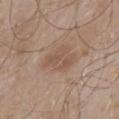<tbp_lesion>
  <biopsy_status>not biopsied; imaged during a skin examination</biopsy_status>
  <automated_metrics>
    <eccentricity>0.75</eccentricity>
    <shape_asymmetry>0.3</shape_asymmetry>
    <border_irregularity_0_10>3.5</border_irregularity_0_10>
    <color_variation_0_10>2.5</color_variation_0_10>
    <peripheral_color_asymmetry>1.0</peripheral_color_asymmetry>
  </automated_metrics>
  <patient>
    <sex>male</sex>
    <age_approx>55</age_approx>
  </patient>
  <site>mid back</site>
  <image>
    <source>total-body photography crop</source>
    <field_of_view_mm>15</field_of_view_mm>
  </image>
  <lesion_size>
    <long_diameter_mm_approx>4.0</long_diameter_mm_approx>
  </lesion_size>
  <lighting>white-light</lighting>
</tbp_lesion>